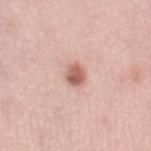biopsy status = imaged on a skin check; not biopsied | patient = female, aged 38–42 | automated metrics = a footprint of about 4 mm², an eccentricity of roughly 0.55, and a symmetry-axis asymmetry near 0.25; an average lesion color of about L≈61 a*≈24 b*≈27 (CIELAB); a nevus-likeness score of about 90/100 | body site = the leg | lesion size = ~2.5 mm (longest diameter) | illumination = white-light illumination | acquisition = 15 mm crop, total-body photography.